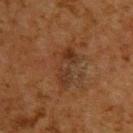No biopsy was performed on this lesion — it was imaged during a full skin examination and was not determined to be concerning.
This image is a 15 mm lesion crop taken from a total-body photograph.
The lesion's longest dimension is about 5 mm.
Located on the upper back.
The lesion-visualizer software estimated an area of roughly 8 mm², an outline eccentricity of about 0.9 (0 = round, 1 = elongated), and a symmetry-axis asymmetry near 0.25. The software also gave a lesion color around L≈28 a*≈17 b*≈26 in CIELAB, a lesion–skin lightness drop of about 6, and a normalized lesion–skin contrast near 6.5. The software also gave a border-irregularity index near 3.5/10 and internal color variation of about 4 on a 0–10 scale.
This is a cross-polarized tile.
The patient is a male roughly 60 years of age.